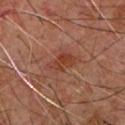No biopsy was performed on this lesion — it was imaged during a full skin examination and was not determined to be concerning.
The tile uses cross-polarized illumination.
This image is a 15 mm lesion crop taken from a total-body photograph.
The lesion is located on the upper back.
A male patient in their 60s.
The recorded lesion diameter is about 3 mm.